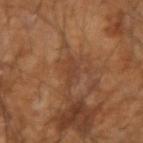follow-up = total-body-photography surveillance lesion; no biopsy
lighting = cross-polarized
patient = male, aged 53 to 57
diameter = ~3.5 mm (longest diameter)
site = the right forearm
acquisition = 15 mm crop, total-body photography